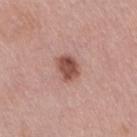workup = imaged on a skin check; not biopsied
patient = female, aged 53 to 57
anatomic site = the right thigh
acquisition = 15 mm crop, total-body photography